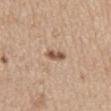{"biopsy_status": "not biopsied; imaged during a skin examination", "lighting": "white-light", "image": {"source": "total-body photography crop", "field_of_view_mm": 15}, "patient": {"sex": "male", "age_approx": 70}, "lesion_size": {"long_diameter_mm_approx": 2.5}, "site": "back"}